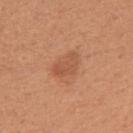biopsy status = imaged on a skin check; not biopsied | TBP lesion metrics = a shape eccentricity near 0.8 and two-axis asymmetry of about 0.3; an average lesion color of about L≈54 a*≈25 b*≈34 (CIELAB), roughly 7 lightness units darker than nearby skin, and a normalized border contrast of about 5; an automated nevus-likeness rating near 30 out of 100 and lesion-presence confidence of about 100/100 | location = the arm | image source = total-body-photography crop, ~15 mm field of view | illumination = white-light | subject = female, aged 38 to 42 | lesion diameter = ≈3.5 mm.